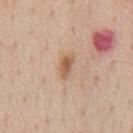Findings:
– workup — imaged on a skin check; not biopsied
– patient — male, roughly 55 years of age
– image-analysis metrics — a lesion area of about 4 mm², an eccentricity of roughly 0.85, and two-axis asymmetry of about 0.2; a mean CIELAB color near L≈59 a*≈19 b*≈32, a lesion–skin lightness drop of about 11, and a lesion-to-skin contrast of about 7.5 (normalized; higher = more distinct); a detector confidence of about 100 out of 100 that the crop contains a lesion
– tile lighting — white-light
– anatomic site — the chest
– acquisition — total-body-photography crop, ~15 mm field of view